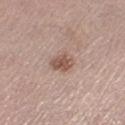workup: no biopsy performed (imaged during a skin exam)
lighting: white-light illumination
TBP lesion metrics: lesion-presence confidence of about 100/100
imaging modality: total-body-photography crop, ~15 mm field of view
site: the right thigh
size: ≈3 mm
patient: female, aged approximately 65